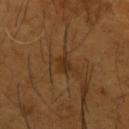About 2.5 mm across. Captured under cross-polarized illumination. A male patient aged around 65. The lesion is on the left forearm. A roughly 15 mm field-of-view crop from a total-body skin photograph.